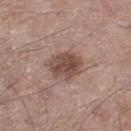Imaged during a routine full-body skin examination; the lesion was not biopsied and no histopathology is available.
A lesion tile, about 15 mm wide, cut from a 3D total-body photograph.
A male patient, in their mid- to late 60s.
From the left thigh.